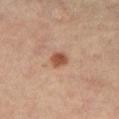The lesion was photographed on a routine skin check and not biopsied; there is no pathology result. The lesion's longest dimension is about 2.5 mm. The subject is a female roughly 65 years of age. A 15 mm close-up extracted from a 3D total-body photography capture. This is a cross-polarized tile. The lesion is on the abdomen.